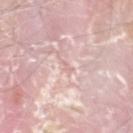Impression: No biopsy was performed on this lesion — it was imaged during a full skin examination and was not determined to be concerning. Background: A male patient, roughly 75 years of age. The lesion is located on the head or neck. Imaged with white-light lighting. A 15 mm close-up extracted from a 3D total-body photography capture. Automated image analysis of the tile measured a footprint of about 1 mm², a shape eccentricity near 0.85, and a symmetry-axis asymmetry near 0.45. It also reported an average lesion color of about L≈71 a*≈20 b*≈22 (CIELAB), a lesion–skin lightness drop of about 6, and a lesion-to-skin contrast of about 4 (normalized; higher = more distinct). Longest diameter approximately 1.5 mm.